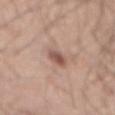No biopsy was performed on this lesion — it was imaged during a full skin examination and was not determined to be concerning. The lesion's longest dimension is about 3.5 mm. Imaged with white-light lighting. A male patient aged around 45. Located on the back. A 15 mm close-up extracted from a 3D total-body photography capture.